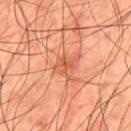Context: From the upper back. A close-up tile cropped from a whole-body skin photograph, about 15 mm across. A male subject, aged 43 to 47.